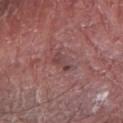biopsy status = catalogued during a skin exam; not biopsied
lesion size = about 3 mm
image = total-body-photography crop, ~15 mm field of view
automated lesion analysis = an area of roughly 3.5 mm², a shape eccentricity near 0.9, and two-axis asymmetry of about 0.45; a nevus-likeness score of about 0/100 and lesion-presence confidence of about 95/100
illumination = white-light illumination
body site = the right forearm
patient = male, aged 68–72